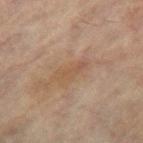Imaged during a routine full-body skin examination; the lesion was not biopsied and no histopathology is available. This is a cross-polarized tile. The lesion is on the leg. Measured at roughly 3.5 mm in maximum diameter. A 15 mm close-up tile from a total-body photography series done for melanoma screening. The patient is a female aged 78–82.